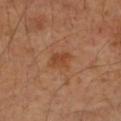  biopsy_status: not biopsied; imaged during a skin examination
  patient:
    sex: female
    age_approx: 60
  automated_metrics:
    area_mm2_approx: 6.0
    eccentricity: 0.7
    shape_asymmetry: 0.3
    cielab_L: 47
    cielab_a: 23
    cielab_b: 36
    vs_skin_darker_L: 7.0
    vs_skin_contrast_norm: 6.0
    nevus_likeness_0_100: 10
    lesion_detection_confidence_0_100: 100
  site: left forearm
  lesion_size:
    long_diameter_mm_approx: 3.0
  image:
    source: total-body photography crop
    field_of_view_mm: 15